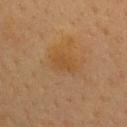imaging modality: 15 mm crop, total-body photography | patient: female, roughly 40 years of age | diameter: about 3 mm | lighting: cross-polarized | location: the upper back.